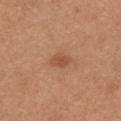Recorded during total-body skin imaging; not selected for excision or biopsy.
The patient is a female roughly 25 years of age.
This is a white-light tile.
A region of skin cropped from a whole-body photographic capture, roughly 15 mm wide.
Located on the right upper arm.
Approximately 2.5 mm at its widest.
The lesion-visualizer software estimated a footprint of about 3.5 mm² and a shape eccentricity near 0.7. It also reported a border-irregularity index near 3/10, a within-lesion color-variation index near 2.5/10, and a peripheral color-asymmetry measure near 1.